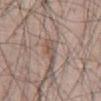Impression:
No biopsy was performed on this lesion — it was imaged during a full skin examination and was not determined to be concerning.
Acquisition and patient details:
Cropped from a total-body skin-imaging series; the visible field is about 15 mm. On the abdomen. A male subject, in their mid- to late 60s.